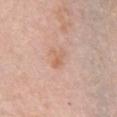Imaged during a routine full-body skin examination; the lesion was not biopsied and no histopathology is available.
The lesion is on the chest.
The lesion-visualizer software estimated a lesion color around L≈63 a*≈21 b*≈31 in CIELAB, roughly 7 lightness units darker than nearby skin, and a lesion-to-skin contrast of about 5.5 (normalized; higher = more distinct). And it measured a border-irregularity rating of about 5/10 and a color-variation rating of about 0.5/10.
The subject is a female aged around 65.
The tile uses white-light illumination.
A roughly 15 mm field-of-view crop from a total-body skin photograph.